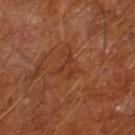patient:
  sex: male
  age_approx: 65
lesion_size:
  long_diameter_mm_approx: 3.0
image:
  source: total-body photography crop
  field_of_view_mm: 15
automated_metrics:
  cielab_L: 34
  cielab_a: 24
  cielab_b: 30
  vs_skin_darker_L: 5.0
  peripheral_color_asymmetry: 0.5
lighting: cross-polarized
site: leg